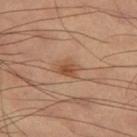biopsy_status: not biopsied; imaged during a skin examination
lighting: cross-polarized
image:
  source: total-body photography crop
  field_of_view_mm: 15
lesion_size:
  long_diameter_mm_approx: 2.5
patient:
  sex: male
  age_approx: 60
site: left thigh